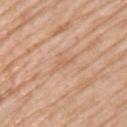Q: Is there a histopathology result?
A: catalogued during a skin exam; not biopsied
Q: What is the anatomic site?
A: the left upper arm
Q: Lesion size?
A: about 3 mm
Q: What did automated image analysis measure?
A: an area of roughly 2.5 mm², a shape eccentricity near 0.95, and a shape-asymmetry score of about 0.5 (0 = symmetric); an automated nevus-likeness rating near 0 out of 100 and a detector confidence of about 70 out of 100 that the crop contains a lesion
Q: What lighting was used for the tile?
A: white-light
Q: How was this image acquired?
A: ~15 mm tile from a whole-body skin photo
Q: Who is the patient?
A: female, approximately 65 years of age